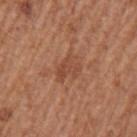Recorded during total-body skin imaging; not selected for excision or biopsy.
From the left upper arm.
This image is a 15 mm lesion crop taken from a total-body photograph.
The tile uses white-light illumination.
Approximately 3.5 mm at its widest.
The lesion-visualizer software estimated an area of roughly 6 mm² and an eccentricity of roughly 0.75. It also reported border irregularity of about 5 on a 0–10 scale, a color-variation rating of about 2/10, and a peripheral color-asymmetry measure near 0.5.
A male subject approximately 65 years of age.